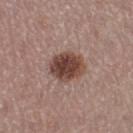notes: imaged on a skin check; not biopsied
site: the left thigh
lighting: white-light illumination
image: ~15 mm crop, total-body skin-cancer survey
lesion size: ~4 mm (longest diameter)
subject: female, in their mid- to late 50s